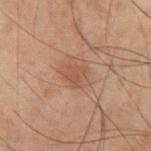Q: Was a biopsy performed?
A: catalogued during a skin exam; not biopsied
Q: Patient demographics?
A: male, in their 70s
Q: What did automated image analysis measure?
A: an average lesion color of about L≈39 a*≈17 b*≈24 (CIELAB), about 5 CIELAB-L* units darker than the surrounding skin, and a normalized lesion–skin contrast near 5; border irregularity of about 3.5 on a 0–10 scale and internal color variation of about 1.5 on a 0–10 scale
Q: What is the imaging modality?
A: total-body-photography crop, ~15 mm field of view
Q: Lesion location?
A: the front of the torso
Q: How large is the lesion?
A: about 2.5 mm